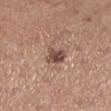Imaged during a routine full-body skin examination; the lesion was not biopsied and no histopathology is available. The tile uses white-light illumination. A lesion tile, about 15 mm wide, cut from a 3D total-body photograph. A female patient aged approximately 60. From the leg.